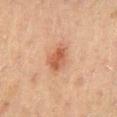Clinical impression: No biopsy was performed on this lesion — it was imaged during a full skin examination and was not determined to be concerning. Context: Imaged with cross-polarized lighting. This image is a 15 mm lesion crop taken from a total-body photograph. The patient is a male about 50 years old. From the back.